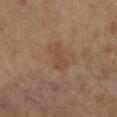Assessment: No biopsy was performed on this lesion — it was imaged during a full skin examination and was not determined to be concerning. Context: A female patient in their mid-60s. From the right leg. This image is a 15 mm lesion crop taken from a total-body photograph. The total-body-photography lesion software estimated internal color variation of about 1 on a 0–10 scale. It also reported an automated nevus-likeness rating near 0 out of 100 and lesion-presence confidence of about 100/100. Longest diameter approximately 3.5 mm.